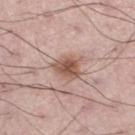Q: Was this lesion biopsied?
A: total-body-photography surveillance lesion; no biopsy
Q: Lesion size?
A: ≈3.5 mm
Q: Lesion location?
A: the left thigh
Q: How was the tile lit?
A: white-light
Q: What are the patient's age and sex?
A: male, approximately 50 years of age
Q: What kind of image is this?
A: ~15 mm tile from a whole-body skin photo
Q: What did automated image analysis measure?
A: an eccentricity of roughly 0.45 and two-axis asymmetry of about 0.3